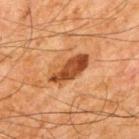workup: total-body-photography surveillance lesion; no biopsy | image source: ~15 mm tile from a whole-body skin photo | body site: the upper back | illumination: cross-polarized | patient: male, in their mid- to late 60s | image-analysis metrics: roughly 12 lightness units darker than nearby skin and a normalized border contrast of about 10; a border-irregularity rating of about 2/10, a within-lesion color-variation index near 5/10, and radial color variation of about 1.5; an automated nevus-likeness rating near 35 out of 100 and a detector confidence of about 100 out of 100 that the crop contains a lesion | diameter: about 5.5 mm.